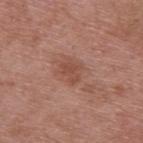Clinical impression: Recorded during total-body skin imaging; not selected for excision or biopsy. Background: The patient is a male about 55 years old. The lesion is located on the upper back. A 15 mm crop from a total-body photograph taken for skin-cancer surveillance. The tile uses white-light illumination. About 2.5 mm across.